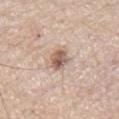biopsy_status: not biopsied; imaged during a skin examination
lesion_size:
  long_diameter_mm_approx: 3.0
patient:
  sex: male
  age_approx: 65
automated_metrics:
  eccentricity: 0.75
  cielab_L: 58
  cielab_a: 17
  cielab_b: 26
  vs_skin_darker_L: 14.0
  border_irregularity_0_10: 2.0
  color_variation_0_10: 5.5
  peripheral_color_asymmetry: 2.0
  nevus_likeness_0_100: 90
site: chest
image:
  source: total-body photography crop
  field_of_view_mm: 15
lighting: white-light